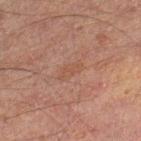No biopsy was performed on this lesion — it was imaged during a full skin examination and was not determined to be concerning. Located on the right lower leg. A male patient, roughly 30 years of age. A roughly 15 mm field-of-view crop from a total-body skin photograph. Longest diameter approximately 3 mm.